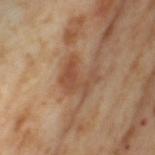| feature | finding |
|---|---|
| location | the left thigh |
| illumination | cross-polarized illumination |
| subject | female, aged around 55 |
| acquisition | total-body-photography crop, ~15 mm field of view |